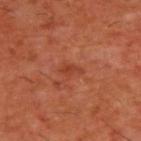– biopsy status · catalogued during a skin exam; not biopsied
– image-analysis metrics · an automated nevus-likeness rating near 0 out of 100 and lesion-presence confidence of about 100/100
– body site · the upper back
– imaging modality · ~15 mm tile from a whole-body skin photo
– patient · male, aged approximately 60
– diameter · ~2.5 mm (longest diameter)